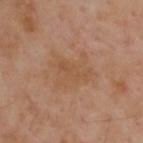| key | value |
|---|---|
| follow-up | total-body-photography surveillance lesion; no biopsy |
| body site | the back |
| illumination | cross-polarized illumination |
| diameter | ≈5 mm |
| imaging modality | total-body-photography crop, ~15 mm field of view |
| patient | male, in their mid-50s |